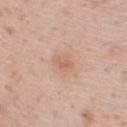Notes:
– workup — imaged on a skin check; not biopsied